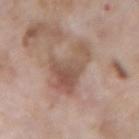workup = no biopsy performed (imaged during a skin exam); illumination = white-light; automated lesion analysis = a shape eccentricity near 0.85 and a symmetry-axis asymmetry near 0.55; patient = male, aged 58–62; lesion size = ~6.5 mm (longest diameter); image source = ~15 mm crop, total-body skin-cancer survey; site = the arm.